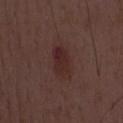The lesion was photographed on a routine skin check and not biopsied; there is no pathology result. This image is a 15 mm lesion crop taken from a total-body photograph. The patient is a male about 50 years old.